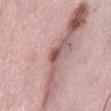Assessment: Recorded during total-body skin imaging; not selected for excision or biopsy. Image and clinical context: An algorithmic analysis of the crop reported a footprint of about 3.5 mm² and two-axis asymmetry of about 0.25. Imaged with white-light lighting. From the abdomen. A 15 mm close-up extracted from a 3D total-body photography capture. The subject is a female aged around 50. Longest diameter approximately 3 mm.